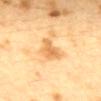Captured during whole-body skin photography for melanoma surveillance; the lesion was not biopsied. The lesion is located on the mid back. A 15 mm crop from a total-body photograph taken for skin-cancer surveillance. The lesion's longest dimension is about 3.5 mm. The lesion-visualizer software estimated an area of roughly 6.5 mm², an eccentricity of roughly 0.6, and two-axis asymmetry of about 0.4. The software also gave roughly 9 lightness units darker than nearby skin and a normalized border contrast of about 6.5. And it measured a border-irregularity index near 4/10 and peripheral color asymmetry of about 0.5. The software also gave a nevus-likeness score of about 0/100. Imaged with cross-polarized lighting. A female subject aged around 40.